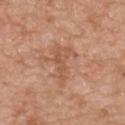Impression:
No biopsy was performed on this lesion — it was imaged during a full skin examination and was not determined to be concerning.
Clinical summary:
The recorded lesion diameter is about 5 mm. Captured under white-light illumination. The total-body-photography lesion software estimated an outline eccentricity of about 0.85 (0 = round, 1 = elongated). And it measured a lesion color around L≈55 a*≈22 b*≈33 in CIELAB and a lesion-to-skin contrast of about 5.5 (normalized; higher = more distinct). The analysis additionally found a classifier nevus-likeness of about 0/100 and lesion-presence confidence of about 100/100. Located on the upper back. A region of skin cropped from a whole-body photographic capture, roughly 15 mm wide. A male patient aged 48–52.